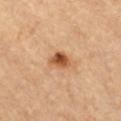Captured during whole-body skin photography for melanoma surveillance; the lesion was not biopsied. The lesion is on the front of the torso. Captured under cross-polarized illumination. Measured at roughly 3 mm in maximum diameter. Automated tile analysis of the lesion measured an area of roughly 4.5 mm², an outline eccentricity of about 0.65 (0 = round, 1 = elongated), and a shape-asymmetry score of about 0.25 (0 = symmetric). The software also gave a lesion color around L≈53 a*≈25 b*≈39 in CIELAB and a lesion–skin lightness drop of about 15. The subject is a female aged 38–42. A 15 mm close-up extracted from a 3D total-body photography capture.